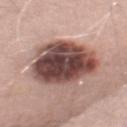biopsy status: total-body-photography surveillance lesion; no biopsy
automated metrics: an average lesion color of about L≈46 a*≈20 b*≈21 (CIELAB) and about 21 CIELAB-L* units darker than the surrounding skin; a classifier nevus-likeness of about 0/100 and a lesion-detection confidence of about 100/100
image: ~15 mm crop, total-body skin-cancer survey
lighting: white-light
diameter: about 8.5 mm
patient: male, aged approximately 65
anatomic site: the back